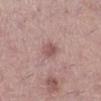{"site": "left lower leg", "lighting": "white-light", "lesion_size": {"long_diameter_mm_approx": 2.5}, "automated_metrics": {"area_mm2_approx": 4.0, "eccentricity": 0.65, "shape_asymmetry": 0.25, "cielab_L": 54, "cielab_a": 22, "cielab_b": 21, "vs_skin_darker_L": 10.0, "vs_skin_contrast_norm": 6.5, "nevus_likeness_0_100": 15, "lesion_detection_confidence_0_100": 100}, "patient": {"sex": "female", "age_approx": 40}, "image": {"source": "total-body photography crop", "field_of_view_mm": 15}}